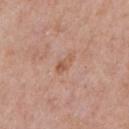Q: Was a biopsy performed?
A: catalogued during a skin exam; not biopsied
Q: Illumination type?
A: white-light illumination
Q: What is the anatomic site?
A: the chest
Q: Lesion size?
A: ≈3 mm
Q: What kind of image is this?
A: ~15 mm tile from a whole-body skin photo
Q: Patient demographics?
A: male, in their 80s
Q: Automated lesion metrics?
A: a lesion color around L≈56 a*≈22 b*≈31 in CIELAB, about 8 CIELAB-L* units darker than the surrounding skin, and a normalized lesion–skin contrast near 6; a border-irregularity index near 3/10, a color-variation rating of about 1.5/10, and peripheral color asymmetry of about 0; an automated nevus-likeness rating near 0 out of 100 and a lesion-detection confidence of about 100/100